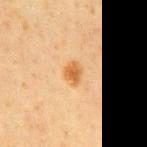Q: Was a biopsy performed?
A: imaged on a skin check; not biopsied
Q: How large is the lesion?
A: about 2.5 mm
Q: What is the imaging modality?
A: ~15 mm tile from a whole-body skin photo
Q: Where on the body is the lesion?
A: the mid back
Q: Patient demographics?
A: male, approximately 60 years of age
Q: Automated lesion metrics?
A: an eccentricity of roughly 0.65 and two-axis asymmetry of about 0.2; a border-irregularity rating of about 2/10, a within-lesion color-variation index near 3/10, and a peripheral color-asymmetry measure near 1; an automated nevus-likeness rating near 95 out of 100
Q: How was the tile lit?
A: cross-polarized illumination